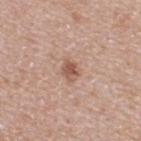– workup: imaged on a skin check; not biopsied
– illumination: white-light
– acquisition: total-body-photography crop, ~15 mm field of view
– subject: female, aged 38–42
– body site: the upper back